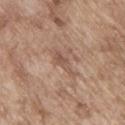biopsy status — total-body-photography surveillance lesion; no biopsy | lighting — white-light illumination | lesion diameter — about 3.5 mm | subject — male, in their mid- to late 60s | location — the upper back | image — ~15 mm tile from a whole-body skin photo.